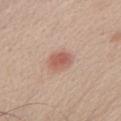<tbp_lesion>
  <biopsy_status>not biopsied; imaged during a skin examination</biopsy_status>
  <lighting>white-light</lighting>
  <image>
    <source>total-body photography crop</source>
    <field_of_view_mm>15</field_of_view_mm>
  </image>
  <site>chest</site>
  <lesion_size>
    <long_diameter_mm_approx>2.5</long_diameter_mm_approx>
  </lesion_size>
  <automated_metrics>
    <eccentricity>0.6</eccentricity>
    <shape_asymmetry>0.15</shape_asymmetry>
  </automated_metrics>
  <patient>
    <sex>male</sex>
    <age_approx>65</age_approx>
  </patient>
</tbp_lesion>